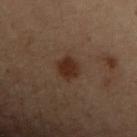  lesion_size:
    long_diameter_mm_approx: 3.0
  image:
    source: total-body photography crop
    field_of_view_mm: 15
  patient:
    sex: female
    age_approx: 55
  site: arm